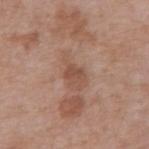  biopsy_status: not biopsied; imaged during a skin examination
  lighting: white-light
  site: abdomen
  automated_metrics:
    area_mm2_approx: 7.0
    cielab_L: 51
    cielab_a: 20
    cielab_b: 28
    vs_skin_darker_L: 8.0
    vs_skin_contrast_norm: 6.0
    lesion_detection_confidence_0_100: 100
  patient:
    sex: male
    age_approx: 70
  lesion_size:
    long_diameter_mm_approx: 4.5
  image:
    source: total-body photography crop
    field_of_view_mm: 15